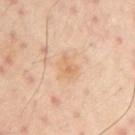biopsy status=total-body-photography surveillance lesion; no biopsy
subject=male, about 50 years old
imaging modality=~15 mm crop, total-body skin-cancer survey
illumination=cross-polarized illumination
site=the left upper arm
size=≈2.5 mm